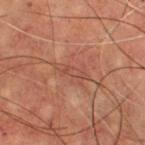– notes · total-body-photography surveillance lesion; no biopsy
– subject · male, in their 70s
– lesion size · ≈3.5 mm
– anatomic site · the chest
– image · 15 mm crop, total-body photography